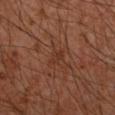Clinical impression:
Recorded during total-body skin imaging; not selected for excision or biopsy.
Acquisition and patient details:
Automated tile analysis of the lesion measured an area of roughly 2.5 mm², an outline eccentricity of about 0.85 (0 = round, 1 = elongated), and two-axis asymmetry of about 0.35. It also reported border irregularity of about 3 on a 0–10 scale, a color-variation rating of about 0.5/10, and radial color variation of about 0. It also reported a lesion-detection confidence of about 95/100. A male patient, aged 53–57. This image is a 15 mm lesion crop taken from a total-body photograph. The lesion is on the right forearm.